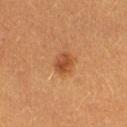Measured at roughly 3 mm in maximum diameter. The lesion-visualizer software estimated a footprint of about 5 mm², an eccentricity of roughly 0.65, and a shape-asymmetry score of about 0.1 (0 = symmetric). The software also gave about 9 CIELAB-L* units darker than the surrounding skin and a normalized border contrast of about 7.5. The analysis additionally found a classifier nevus-likeness of about 90/100 and lesion-presence confidence of about 100/100. A female patient, approximately 40 years of age. On the right thigh. This image is a 15 mm lesion crop taken from a total-body photograph.